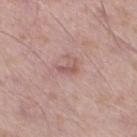Captured during whole-body skin photography for melanoma surveillance; the lesion was not biopsied.